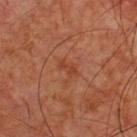subject: male, aged 63–67 | imaging modality: total-body-photography crop, ~15 mm field of view | location: the chest | lesion diameter: ~2.5 mm (longest diameter) | automated lesion analysis: an eccentricity of roughly 0.85 and a shape-asymmetry score of about 0.35 (0 = symmetric); about 6 CIELAB-L* units darker than the surrounding skin and a normalized border contrast of about 5.5; a border-irregularity index near 4/10 and radial color variation of about 0; a nevus-likeness score of about 0/100 and lesion-presence confidence of about 100/100.